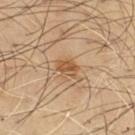Impression:
Part of a total-body skin-imaging series; this lesion was reviewed on a skin check and was not flagged for biopsy.
Background:
The recorded lesion diameter is about 2.5 mm. Automated tile analysis of the lesion measured an area of roughly 4 mm² and a shape eccentricity near 0.7. It also reported internal color variation of about 2.5 on a 0–10 scale and radial color variation of about 1. On the chest. A male subject, aged around 55. Imaged with cross-polarized lighting. A roughly 15 mm field-of-view crop from a total-body skin photograph.